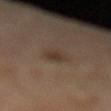Notes:
• notes — total-body-photography surveillance lesion; no biopsy
• subject — female, about 60 years old
• location — the left lower leg
• imaging modality — ~15 mm crop, total-body skin-cancer survey
• lesion diameter — about 3 mm